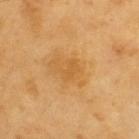Notes:
- follow-up · no biopsy performed (imaged during a skin exam)
- subject · male, aged around 60
- automated metrics · a mean CIELAB color near L≈57 a*≈22 b*≈47 and roughly 6 lightness units darker than nearby skin; a nevus-likeness score of about 10/100 and a lesion-detection confidence of about 100/100
- lesion diameter · about 2.5 mm
- image · ~15 mm tile from a whole-body skin photo
- site · the upper back